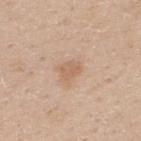{"biopsy_status": "not biopsied; imaged during a skin examination", "site": "upper back", "automated_metrics": {"cielab_L": 62, "cielab_a": 18, "cielab_b": 32, "vs_skin_darker_L": 8.0, "vs_skin_contrast_norm": 5.5, "border_irregularity_0_10": 3.0, "color_variation_0_10": 1.5, "peripheral_color_asymmetry": 0.5}, "patient": {"sex": "male", "age_approx": 30}, "image": {"source": "total-body photography crop", "field_of_view_mm": 15}, "lighting": "white-light"}